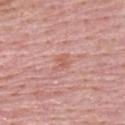Part of a total-body skin-imaging series; this lesion was reviewed on a skin check and was not flagged for biopsy.
Located on the upper back.
A male patient, in their mid-60s.
Approximately 2.5 mm at its widest.
A 15 mm close-up extracted from a 3D total-body photography capture.